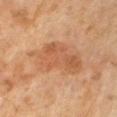biopsy status = imaged on a skin check; not biopsied
lighting = cross-polarized
acquisition = ~15 mm tile from a whole-body skin photo
patient = male, about 65 years old